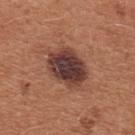Recorded during total-body skin imaging; not selected for excision or biopsy. This image is a 15 mm lesion crop taken from a total-body photograph. A female subject aged around 35. On the chest. Imaged with white-light lighting. Approximately 5 mm at its widest.